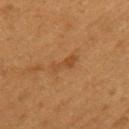  biopsy_status: not biopsied; imaged during a skin examination
  site: left upper arm
  image:
    source: total-body photography crop
    field_of_view_mm: 15
  lesion_size:
    long_diameter_mm_approx: 3.5
  lighting: cross-polarized
  patient:
    sex: male
    age_approx: 55